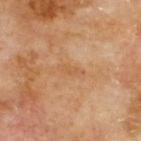lesion diameter=about 3 mm | subject=male, aged around 70 | image source=total-body-photography crop, ~15 mm field of view | body site=the upper back | image-analysis metrics=an area of roughly 3 mm², an eccentricity of roughly 0.9, and a symmetry-axis asymmetry near 0.25; a nevus-likeness score of about 0/100 and lesion-presence confidence of about 100/100.